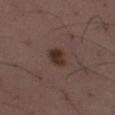The lesion is on the leg.
A close-up tile cropped from a whole-body skin photograph, about 15 mm across.
The total-body-photography lesion software estimated a lesion area of about 4.5 mm². The analysis additionally found an average lesion color of about L≈31 a*≈17 b*≈21 (CIELAB), roughly 10 lightness units darker than nearby skin, and a normalized lesion–skin contrast near 10. The analysis additionally found a border-irregularity rating of about 2/10, a within-lesion color-variation index near 3/10, and radial color variation of about 1.
The recorded lesion diameter is about 2.5 mm.
A male patient, in their 40s.
The tile uses white-light illumination.